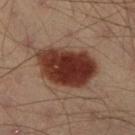Assessment: Captured during whole-body skin photography for melanoma surveillance; the lesion was not biopsied. Image and clinical context: A male patient, aged approximately 40. The lesion is on the right lower leg. An algorithmic analysis of the crop reported a mean CIELAB color near L≈29 a*≈21 b*≈23, a lesion–skin lightness drop of about 16, and a lesion-to-skin contrast of about 14.5 (normalized; higher = more distinct). The software also gave a border-irregularity index near 2.5/10, a color-variation rating of about 4.5/10, and radial color variation of about 1.5. Captured under cross-polarized illumination. This image is a 15 mm lesion crop taken from a total-body photograph. The lesion's longest dimension is about 7 mm.